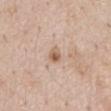Q: Is there a histopathology result?
A: imaged on a skin check; not biopsied
Q: Lesion size?
A: about 2.5 mm
Q: Patient demographics?
A: male, aged around 60
Q: How was this image acquired?
A: ~15 mm crop, total-body skin-cancer survey
Q: What lighting was used for the tile?
A: white-light
Q: What is the anatomic site?
A: the chest